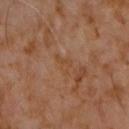  biopsy_status: not biopsied; imaged during a skin examination
  automated_metrics:
    vs_skin_contrast_norm: 4.5
  lesion_size:
    long_diameter_mm_approx: 2.5
  patient:
    sex: male
    age_approx: 60
  image:
    source: total-body photography crop
    field_of_view_mm: 15
  lighting: cross-polarized
  site: chest